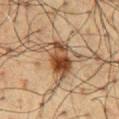Case summary:
– subject — male, aged 58 to 62
– image source — total-body-photography crop, ~15 mm field of view
– tile lighting — cross-polarized
– anatomic site — the chest
– lesion diameter — ~5.5 mm (longest diameter)
– image-analysis metrics — an area of roughly 12 mm², an eccentricity of roughly 0.85, and a symmetry-axis asymmetry near 0.3; border irregularity of about 3.5 on a 0–10 scale and internal color variation of about 9 on a 0–10 scale